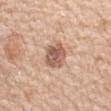Notes:
– workup — catalogued during a skin exam; not biopsied
– patient — female, aged approximately 45
– illumination — white-light illumination
– location — the left forearm
– size — ≈3 mm
– automated metrics — a border-irregularity rating of about 1.5/10, a color-variation rating of about 4/10, and peripheral color asymmetry of about 1; a nevus-likeness score of about 30/100 and lesion-presence confidence of about 100/100
– image — ~15 mm crop, total-body skin-cancer survey